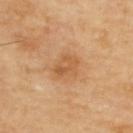On the upper back. Approximately 3.5 mm at its widest. This is a cross-polarized tile. A female subject, aged 43–47. A region of skin cropped from a whole-body photographic capture, roughly 15 mm wide.